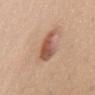No biopsy was performed on this lesion — it was imaged during a full skin examination and was not determined to be concerning. The lesion-visualizer software estimated a lesion area of about 9 mm², an eccentricity of roughly 0.8, and two-axis asymmetry of about 0.3. It also reported an average lesion color of about L≈55 a*≈22 b*≈30 (CIELAB), about 13 CIELAB-L* units darker than the surrounding skin, and a normalized lesion–skin contrast near 8.5. It also reported a classifier nevus-likeness of about 95/100 and a detector confidence of about 100 out of 100 that the crop contains a lesion. About 4.5 mm across. A female patient aged 23 to 27. The lesion is located on the arm. This is a white-light tile. A lesion tile, about 15 mm wide, cut from a 3D total-body photograph.